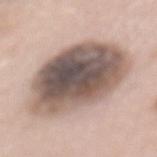Recorded during total-body skin imaging; not selected for excision or biopsy.
A female subject in their 60s.
The lesion is located on the mid back.
An algorithmic analysis of the crop reported a footprint of about 50 mm² and a symmetry-axis asymmetry near 0.15. The analysis additionally found a lesion color around L≈54 a*≈14 b*≈21 in CIELAB, roughly 19 lightness units darker than nearby skin, and a normalized border contrast of about 12.5. The analysis additionally found border irregularity of about 1.5 on a 0–10 scale and radial color variation of about 2.5. And it measured a classifier nevus-likeness of about 0/100 and lesion-presence confidence of about 95/100.
A region of skin cropped from a whole-body photographic capture, roughly 15 mm wide.
Longest diameter approximately 10 mm.
This is a white-light tile.